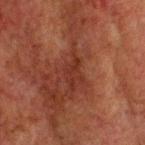  biopsy_status: not biopsied; imaged during a skin examination
  lighting: cross-polarized
  site: head or neck
  image:
    source: total-body photography crop
    field_of_view_mm: 15
  lesion_size:
    long_diameter_mm_approx: 3.5
  patient:
    sex: male
    age_approx: 60
  automated_metrics:
    area_mm2_approx: 3.5
    eccentricity: 0.95
    shape_asymmetry: 0.6
    nevus_likeness_0_100: 0